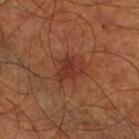| feature | finding |
|---|---|
| follow-up | total-body-photography surveillance lesion; no biopsy |
| body site | the right lower leg |
| lighting | cross-polarized |
| patient | approximately 65 years of age |
| image | total-body-photography crop, ~15 mm field of view |